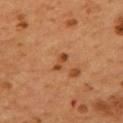anatomic site: the mid back | size: ≈2.5 mm | patient: male, aged 53–57 | image source: ~15 mm crop, total-body skin-cancer survey | illumination: cross-polarized illumination | automated lesion analysis: a lesion color around L≈42 a*≈24 b*≈36 in CIELAB, about 10 CIELAB-L* units darker than the surrounding skin, and a normalized border contrast of about 8; a within-lesion color-variation index near 0/10 and peripheral color asymmetry of about 0.